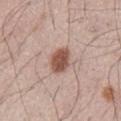Part of a total-body skin-imaging series; this lesion was reviewed on a skin check and was not flagged for biopsy.